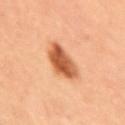This lesion was catalogued during total-body skin photography and was not selected for biopsy. Located on the right upper arm. A female subject roughly 35 years of age. A region of skin cropped from a whole-body photographic capture, roughly 15 mm wide.